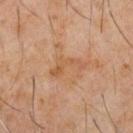biopsy status: no biopsy performed (imaged during a skin exam) | diameter: ≈4.5 mm | imaging modality: 15 mm crop, total-body photography | subject: male, aged 58 to 62 | body site: the chest.